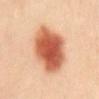Part of a total-body skin-imaging series; this lesion was reviewed on a skin check and was not flagged for biopsy. This image is a 15 mm lesion crop taken from a total-body photograph. Longest diameter approximately 7 mm. This is a cross-polarized tile. A female subject, in their mid-30s. The lesion is located on the chest. Automated tile analysis of the lesion measured roughly 19 lightness units darker than nearby skin and a lesion-to-skin contrast of about 11.5 (normalized; higher = more distinct). The software also gave a border-irregularity rating of about 1.5/10, a color-variation rating of about 6.5/10, and a peripheral color-asymmetry measure near 1.5.